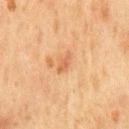| field | value |
|---|---|
| biopsy status | imaged on a skin check; not biopsied |
| site | the chest |
| image source | ~15 mm crop, total-body skin-cancer survey |
| patient | male, aged around 75 |